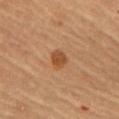workup: total-body-photography surveillance lesion; no biopsy
automated lesion analysis: a footprint of about 4.5 mm², an eccentricity of roughly 0.55, and two-axis asymmetry of about 0.25; an average lesion color of about L≈46 a*≈22 b*≈35 (CIELAB), a lesion–skin lightness drop of about 9, and a lesion-to-skin contrast of about 7.5 (normalized; higher = more distinct)
imaging modality: ~15 mm crop, total-body skin-cancer survey
patient: female, in their 60s
tile lighting: cross-polarized illumination
size: about 2.5 mm
site: the front of the torso